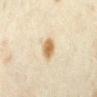{
  "biopsy_status": "not biopsied; imaged during a skin examination",
  "lesion_size": {
    "long_diameter_mm_approx": 2.5
  },
  "patient": {
    "sex": "female",
    "age_approx": 35
  },
  "image": {
    "source": "total-body photography crop",
    "field_of_view_mm": 15
  },
  "site": "right forearm"
}